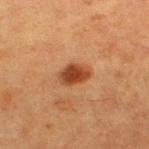Case summary:
– follow-up — imaged on a skin check; not biopsied
– imaging modality — 15 mm crop, total-body photography
– patient — female, roughly 50 years of age
– body site — the left thigh